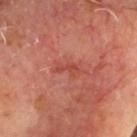A male patient roughly 60 years of age.
The tile uses cross-polarized illumination.
This image is a 15 mm lesion crop taken from a total-body photograph.
Located on the head or neck.
Longest diameter approximately 3 mm.
An algorithmic analysis of the crop reported an eccentricity of roughly 0.9 and a shape-asymmetry score of about 0.35 (0 = symmetric).